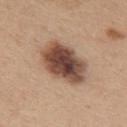  biopsy_status: not biopsied; imaged during a skin examination
  image:
    source: total-body photography crop
    field_of_view_mm: 15
  patient:
    sex: female
    age_approx: 45
  site: upper back